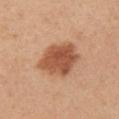Background:
The total-body-photography lesion software estimated a footprint of about 17 mm² and a shape-asymmetry score of about 0.2 (0 = symmetric). A 15 mm crop from a total-body photograph taken for skin-cancer surveillance. Captured under white-light illumination. Measured at roughly 5 mm in maximum diameter. The lesion is located on the left upper arm. A female patient in their mid- to late 40s.